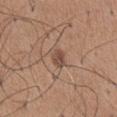Acquisition and patient details: The lesion is located on the chest. This is a white-light tile. A 15 mm close-up tile from a total-body photography series done for melanoma screening. An algorithmic analysis of the crop reported a lesion area of about 3.5 mm², an outline eccentricity of about 0.75 (0 = round, 1 = elongated), and a symmetry-axis asymmetry near 0.25. It also reported a border-irregularity index near 2.5/10 and internal color variation of about 4 on a 0–10 scale. A male subject in their mid- to late 60s. Longest diameter approximately 2.5 mm.